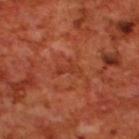| key | value |
|---|---|
| biopsy status | catalogued during a skin exam; not biopsied |
| lesion diameter | ≈3 mm |
| subject | male, aged 68–72 |
| illumination | cross-polarized |
| body site | the back |
| image | ~15 mm crop, total-body skin-cancer survey |